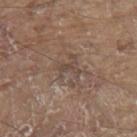Case summary:
- workup: imaged on a skin check; not biopsied
- patient: male, roughly 80 years of age
- illumination: white-light
- body site: the upper back
- acquisition: 15 mm crop, total-body photography
- lesion diameter: about 2.5 mm